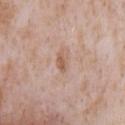Part of a total-body skin-imaging series; this lesion was reviewed on a skin check and was not flagged for biopsy. A male subject aged around 65. On the chest. Cropped from a total-body skin-imaging series; the visible field is about 15 mm.